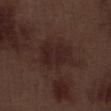This lesion was catalogued during total-body skin photography and was not selected for biopsy. A 15 mm close-up extracted from a 3D total-body photography capture. Captured under white-light illumination. The lesion is on the right lower leg. The recorded lesion diameter is about 4.5 mm. A male patient aged 68 to 72.